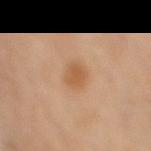Captured during whole-body skin photography for melanoma surveillance; the lesion was not biopsied.
Cropped from a total-body skin-imaging series; the visible field is about 15 mm.
The lesion is located on the right thigh.
Longest diameter approximately 3 mm.
A female patient, aged 48–52.